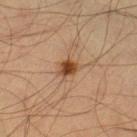The lesion was tiled from a total-body skin photograph and was not biopsied. Located on the leg. The patient is a male in their mid-30s. Cropped from a whole-body photographic skin survey; the tile spans about 15 mm. This is a cross-polarized tile.